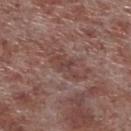follow-up: total-body-photography surveillance lesion; no biopsy | patient: male, aged 53–57 | lesion size: ~3.5 mm (longest diameter) | lighting: white-light | imaging modality: total-body-photography crop, ~15 mm field of view | location: the left lower leg | automated metrics: a lesion area of about 4.5 mm², a shape eccentricity near 0.85, and a symmetry-axis asymmetry near 0.45; border irregularity of about 6 on a 0–10 scale and a peripheral color-asymmetry measure near 0.5; an automated nevus-likeness rating near 0 out of 100 and lesion-presence confidence of about 90/100.